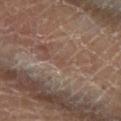Captured during whole-body skin photography for melanoma surveillance; the lesion was not biopsied.
A male subject, aged 58 to 62.
Automated tile analysis of the lesion measured a footprint of about 1 mm², an outline eccentricity of about 0.7 (0 = round, 1 = elongated), and a shape-asymmetry score of about 0.35 (0 = symmetric). It also reported a lesion–skin lightness drop of about 4 and a normalized lesion–skin contrast near 4. The analysis additionally found border irregularity of about 3 on a 0–10 scale, internal color variation of about 0 on a 0–10 scale, and radial color variation of about 0. The software also gave a nevus-likeness score of about 0/100 and lesion-presence confidence of about 35/100.
A 15 mm crop from a total-body photograph taken for skin-cancer surveillance.
Imaged with cross-polarized lighting.
The lesion is on the left lower leg.
Measured at roughly 1 mm in maximum diameter.